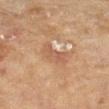workup = total-body-photography surveillance lesion; no biopsy
lesion size = ~4 mm (longest diameter)
subject = female, aged around 80
body site = the left forearm
illumination = cross-polarized
imaging modality = total-body-photography crop, ~15 mm field of view